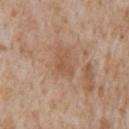The lesion was tiled from a total-body skin photograph and was not biopsied.